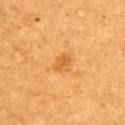Notes:
* workup — no biopsy performed (imaged during a skin exam)
* image — total-body-photography crop, ~15 mm field of view
* anatomic site — the back
* illumination — cross-polarized
* subject — female, aged 48–52